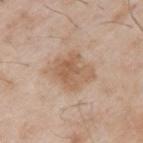Clinical impression: Recorded during total-body skin imaging; not selected for excision or biopsy. Clinical summary: The lesion is on the left upper arm. The patient is a male about 65 years old. Approximately 5 mm at its widest. A region of skin cropped from a whole-body photographic capture, roughly 15 mm wide. The tile uses white-light illumination.